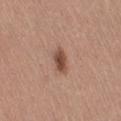<lesion>
<biopsy_status>not biopsied; imaged during a skin examination</biopsy_status>
<patient>
  <sex>female</sex>
  <age_approx>50</age_approx>
</patient>
<automated_metrics>
  <cielab_L>49</cielab_L>
  <cielab_a>21</cielab_a>
  <cielab_b>27</cielab_b>
  <vs_skin_darker_L>12.0</vs_skin_darker_L>
  <vs_skin_contrast_norm>9.0</vs_skin_contrast_norm>
  <color_variation_0_10>3.5</color_variation_0_10>
  <peripheral_color_asymmetry>1.0</peripheral_color_asymmetry>
</automated_metrics>
<site>right thigh</site>
<lighting>white-light</lighting>
<image>
  <source>total-body photography crop</source>
  <field_of_view_mm>15</field_of_view_mm>
</image>
</lesion>